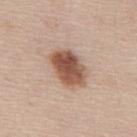biopsy status: catalogued during a skin exam; not biopsied
location: the mid back
image source: ~15 mm tile from a whole-body skin photo
lesion diameter: ≈5 mm
image-analysis metrics: an outline eccentricity of about 0.75 (0 = round, 1 = elongated) and two-axis asymmetry of about 0.15; roughly 16 lightness units darker than nearby skin and a normalized lesion–skin contrast near 10.5; border irregularity of about 1.5 on a 0–10 scale and a color-variation rating of about 5.5/10; an automated nevus-likeness rating near 100 out of 100 and a lesion-detection confidence of about 100/100
lighting: white-light illumination
patient: male, roughly 45 years of age